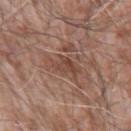The lesion was tiled from a total-body skin photograph and was not biopsied. A male patient roughly 75 years of age. The lesion's longest dimension is about 4.5 mm. Automated tile analysis of the lesion measured a border-irregularity rating of about 5.5/10, a color-variation rating of about 4.5/10, and radial color variation of about 1.5. It also reported lesion-presence confidence of about 100/100. Cropped from a whole-body photographic skin survey; the tile spans about 15 mm. Captured under white-light illumination. From the left upper arm.